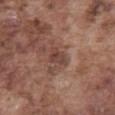{"biopsy_status": "not biopsied; imaged during a skin examination", "image": {"source": "total-body photography crop", "field_of_view_mm": 15}, "lesion_size": {"long_diameter_mm_approx": 2.5}, "site": "abdomen", "automated_metrics": {"area_mm2_approx": 3.5, "shape_asymmetry": 0.3, "cielab_L": 42, "cielab_a": 21, "cielab_b": 23, "vs_skin_contrast_norm": 7.0, "border_irregularity_0_10": 3.0, "color_variation_0_10": 3.5, "peripheral_color_asymmetry": 1.5, "lesion_detection_confidence_0_100": 100}, "patient": {"sex": "male", "age_approx": 75}}